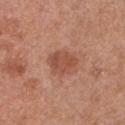Clinical impression: Imaged during a routine full-body skin examination; the lesion was not biopsied and no histopathology is available. Acquisition and patient details: About 3.5 mm across. A 15 mm close-up extracted from a 3D total-body photography capture. An algorithmic analysis of the crop reported an area of roughly 8.5 mm², an outline eccentricity of about 0.6 (0 = round, 1 = elongated), and two-axis asymmetry of about 0.2. It also reported a nevus-likeness score of about 40/100. A female subject, aged 48 to 52. On the chest.